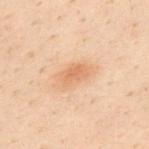Located on the back.
The subject is a male approximately 30 years of age.
A 15 mm crop from a total-body photograph taken for skin-cancer surveillance.